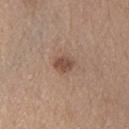The lesion was tiled from a total-body skin photograph and was not biopsied. Approximately 2.5 mm at its widest. This image is a 15 mm lesion crop taken from a total-body photograph. An algorithmic analysis of the crop reported a footprint of about 4.5 mm², a shape eccentricity near 0.6, and a symmetry-axis asymmetry near 0.2. The analysis additionally found roughly 11 lightness units darker than nearby skin and a lesion-to-skin contrast of about 8 (normalized; higher = more distinct). And it measured a border-irregularity rating of about 2/10, internal color variation of about 2.5 on a 0–10 scale, and radial color variation of about 1. The lesion is located on the right upper arm. The tile uses white-light illumination. The subject is a female aged 63–67.